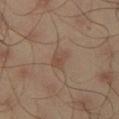Clinical impression:
The lesion was photographed on a routine skin check and not biopsied; there is no pathology result.
Background:
An algorithmic analysis of the crop reported a lesion color around L≈46 a*≈16 b*≈26 in CIELAB, roughly 6 lightness units darker than nearby skin, and a normalized lesion–skin contrast near 5. The software also gave a classifier nevus-likeness of about 10/100 and a lesion-detection confidence of about 100/100. The lesion is on the right thigh. A male subject, in their mid- to late 40s. The lesion's longest dimension is about 2.5 mm. A 15 mm crop from a total-body photograph taken for skin-cancer surveillance.